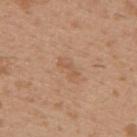workup = total-body-photography surveillance lesion; no biopsy
anatomic site = the upper back
lesion diameter = about 3 mm
imaging modality = 15 mm crop, total-body photography
image-analysis metrics = a border-irregularity rating of about 5.5/10, internal color variation of about 0 on a 0–10 scale, and a peripheral color-asymmetry measure near 0; a lesion-detection confidence of about 100/100
patient = male, in their 30s